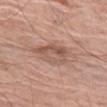Notes:
* notes · no biopsy performed (imaged during a skin exam)
* lesion diameter · ~4.5 mm (longest diameter)
* subject · female, approximately 75 years of age
* location · the left thigh
* automated metrics · an area of roughly 10 mm², a shape eccentricity near 0.65, and two-axis asymmetry of about 0.3; roughly 9 lightness units darker than nearby skin
* tile lighting · white-light
* imaging modality · ~15 mm tile from a whole-body skin photo